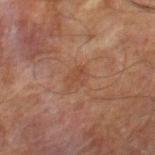Findings:
* follow-up · total-body-photography surveillance lesion; no biopsy
* location · the right thigh
* image source · ~15 mm tile from a whole-body skin photo
* automated lesion analysis · a lesion area of about 3.5 mm² and two-axis asymmetry of about 0.35
* tile lighting · cross-polarized illumination
* patient · male, in their 70s
* size · ≈3 mm